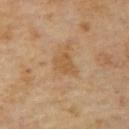{"image": {"source": "total-body photography crop", "field_of_view_mm": 15}, "lighting": "cross-polarized", "automated_metrics": {"cielab_L": 53, "cielab_a": 18, "cielab_b": 36, "vs_skin_darker_L": 7.0, "nevus_likeness_0_100": 5, "lesion_detection_confidence_0_100": 100}, "patient": {"sex": "male", "age_approx": 65}, "lesion_size": {"long_diameter_mm_approx": 3.0}}